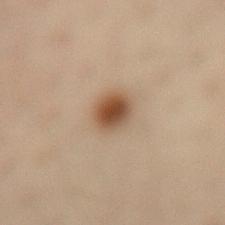Case summary:
– follow-up · total-body-photography surveillance lesion; no biopsy
– image-analysis metrics · a lesion area of about 6 mm², a shape eccentricity near 0.35, and two-axis asymmetry of about 0.1; an average lesion color of about L≈41 a*≈15 b*≈27 (CIELAB), about 12 CIELAB-L* units darker than the surrounding skin, and a normalized border contrast of about 10.5; a border-irregularity index near 1/10, a color-variation rating of about 5/10, and peripheral color asymmetry of about 1.5
– body site · the lower back
– patient · female, roughly 55 years of age
– imaging modality · ~15 mm tile from a whole-body skin photo
– lesion diameter · ≈3 mm
– tile lighting · cross-polarized illumination